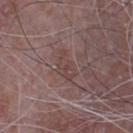{
  "image": {
    "source": "total-body photography crop",
    "field_of_view_mm": 15
  },
  "site": "chest",
  "patient": {
    "sex": "male",
    "age_approx": 65
  }
}